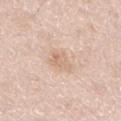Case summary:
• workup: catalogued during a skin exam; not biopsied
• TBP lesion metrics: border irregularity of about 3 on a 0–10 scale, a within-lesion color-variation index near 3/10, and peripheral color asymmetry of about 1; a nevus-likeness score of about 0/100 and a detector confidence of about 100 out of 100 that the crop contains a lesion
• patient: female, about 45 years old
• lesion size: ≈3 mm
• lighting: white-light
• image source: 15 mm crop, total-body photography
• body site: the left lower leg